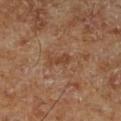A 15 mm crop from a total-body photograph taken for skin-cancer surveillance. The subject is a male aged approximately 70. Located on the right lower leg. This is a cross-polarized tile. Longest diameter approximately 2.5 mm.